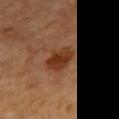Recorded during total-body skin imaging; not selected for excision or biopsy. Automated tile analysis of the lesion measured a shape eccentricity near 0.8. The software also gave an average lesion color of about L≈36 a*≈24 b*≈33 (CIELAB), about 10 CIELAB-L* units darker than the surrounding skin, and a normalized border contrast of about 9.5. The software also gave a border-irregularity rating of about 2/10, a within-lesion color-variation index near 4/10, and peripheral color asymmetry of about 1.5. Imaged with cross-polarized lighting. A roughly 15 mm field-of-view crop from a total-body skin photograph. A male patient, aged 63–67. The lesion is on the mid back.